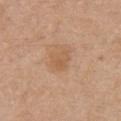<tbp_lesion>
  <biopsy_status>not biopsied; imaged during a skin examination</biopsy_status>
  <lesion_size>
    <long_diameter_mm_approx>2.5</long_diameter_mm_approx>
  </lesion_size>
  <patient>
    <sex>male</sex>
    <age_approx>70</age_approx>
  </patient>
  <site>chest</site>
  <image>
    <source>total-body photography crop</source>
    <field_of_view_mm>15</field_of_view_mm>
  </image>
  <lighting>white-light</lighting>
  <automated_metrics>
    <cielab_L>58</cielab_L>
    <cielab_a>20</cielab_a>
    <cielab_b>35</cielab_b>
  </automated_metrics>
</tbp_lesion>